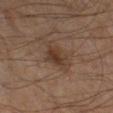Captured during whole-body skin photography for melanoma surveillance; the lesion was not biopsied. Automated image analysis of the tile measured a lesion area of about 7.5 mm² and a shape eccentricity near 0.65. The software also gave a mean CIELAB color near L≈34 a*≈15 b*≈24, about 7 CIELAB-L* units darker than the surrounding skin, and a normalized lesion–skin contrast near 7. The analysis additionally found a border-irregularity rating of about 3.5/10 and peripheral color asymmetry of about 1. It also reported an automated nevus-likeness rating near 55 out of 100. Located on the right lower leg. Captured under cross-polarized illumination. This image is a 15 mm lesion crop taken from a total-body photograph. About 3.5 mm across. A male subject aged around 55.